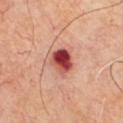Imaged during a routine full-body skin examination; the lesion was not biopsied and no histopathology is available. Captured under cross-polarized illumination. The lesion-visualizer software estimated an average lesion color of about L≈47 a*≈34 b*≈27 (CIELAB), roughly 20 lightness units darker than nearby skin, and a normalized lesion–skin contrast near 13.5. The software also gave an automated nevus-likeness rating near 0 out of 100. A region of skin cropped from a whole-body photographic capture, roughly 15 mm wide. The subject is aged around 65. Measured at roughly 3.5 mm in maximum diameter. From the chest.